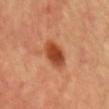biopsy status: no biopsy performed (imaged during a skin exam)
imaging modality: total-body-photography crop, ~15 mm field of view
size: ~4 mm (longest diameter)
automated lesion analysis: a lesion area of about 8.5 mm², an outline eccentricity of about 0.8 (0 = round, 1 = elongated), and a symmetry-axis asymmetry near 0.15; a within-lesion color-variation index near 4/10 and radial color variation of about 1; an automated nevus-likeness rating near 100 out of 100 and a lesion-detection confidence of about 100/100
anatomic site: the chest
patient: male, in their mid- to late 50s
lighting: cross-polarized